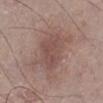Q: Was a biopsy performed?
A: catalogued during a skin exam; not biopsied
Q: Automated lesion metrics?
A: a lesion area of about 11 mm², an outline eccentricity of about 0.7 (0 = round, 1 = elongated), and a shape-asymmetry score of about 0.35 (0 = symmetric)
Q: What is the lesion's diameter?
A: ≈4.5 mm
Q: How was this image acquired?
A: total-body-photography crop, ~15 mm field of view
Q: What lighting was used for the tile?
A: white-light illumination
Q: Patient demographics?
A: male, aged approximately 50
Q: What is the anatomic site?
A: the leg